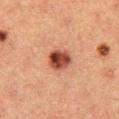Imaged during a routine full-body skin examination; the lesion was not biopsied and no histopathology is available. Approximately 3 mm at its widest. Cropped from a whole-body photographic skin survey; the tile spans about 15 mm. On the left thigh. The subject is a female about 40 years old. Captured under cross-polarized illumination.